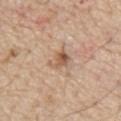biopsy_status: not biopsied; imaged during a skin examination
patient:
  sex: male
  age_approx: 70
lighting: white-light
image:
  source: total-body photography crop
  field_of_view_mm: 15
site: front of the torso